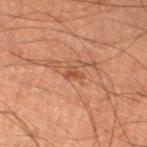A male subject roughly 60 years of age. The lesion is on the left thigh. This image is a 15 mm lesion crop taken from a total-body photograph.